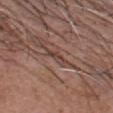Notes:
• biopsy status — total-body-photography surveillance lesion; no biopsy
• imaging modality — ~15 mm crop, total-body skin-cancer survey
• body site — the head or neck
• tile lighting — white-light
• subject — male, approximately 55 years of age